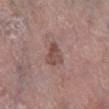follow-up = catalogued during a skin exam; not biopsied
patient = male, aged approximately 70
automated lesion analysis = about 9 CIELAB-L* units darker than the surrounding skin and a normalized lesion–skin contrast near 7; border irregularity of about 3 on a 0–10 scale, a within-lesion color-variation index near 3.5/10, and peripheral color asymmetry of about 1.5
lighting = white-light
location = the right lower leg
lesion size = ≈2.5 mm
image = ~15 mm tile from a whole-body skin photo